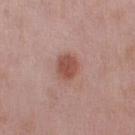Clinical impression:
Imaged during a routine full-body skin examination; the lesion was not biopsied and no histopathology is available.
Acquisition and patient details:
The lesion is located on the right upper arm. Cropped from a whole-body photographic skin survey; the tile spans about 15 mm. Longest diameter approximately 3 mm. A male subject about 55 years old. The total-body-photography lesion software estimated a lesion area of about 6.5 mm², a shape eccentricity near 0.55, and a symmetry-axis asymmetry near 0.15. Captured under white-light illumination.